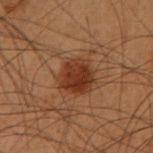Q: Was this lesion biopsied?
A: total-body-photography surveillance lesion; no biopsy
Q: Who is the patient?
A: male, about 55 years old
Q: Automated lesion metrics?
A: a footprint of about 11 mm², an eccentricity of roughly 0.3, and a symmetry-axis asymmetry near 0.2; an average lesion color of about L≈28 a*≈20 b*≈27 (CIELAB), a lesion–skin lightness drop of about 9, and a normalized lesion–skin contrast near 9; a peripheral color-asymmetry measure near 1; a nevus-likeness score of about 95/100 and a detector confidence of about 100 out of 100 that the crop contains a lesion
Q: Lesion size?
A: about 4 mm
Q: Lesion location?
A: the arm
Q: What kind of image is this?
A: ~15 mm tile from a whole-body skin photo